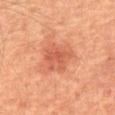Notes:
* workup: catalogued during a skin exam; not biopsied
* acquisition: 15 mm crop, total-body photography
* lesion diameter: ~4 mm (longest diameter)
* anatomic site: the abdomen
* patient: male, aged around 65